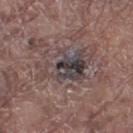Notes:
• notes: imaged on a skin check; not biopsied
• lesion diameter: about 6.5 mm
• automated lesion analysis: an area of roughly 16 mm² and an outline eccentricity of about 0.85 (0 = round, 1 = elongated); a normalized border contrast of about 9.5; a border-irregularity rating of about 8/10, a color-variation rating of about 10/10, and peripheral color asymmetry of about 3.5; a lesion-detection confidence of about 65/100
• acquisition: total-body-photography crop, ~15 mm field of view
• location: the right thigh
• patient: male, aged approximately 80